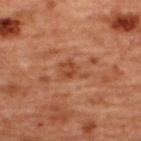Imaged during a routine full-body skin examination; the lesion was not biopsied and no histopathology is available. Measured at roughly 3.5 mm in maximum diameter. Cropped from a whole-body photographic skin survey; the tile spans about 15 mm. On the upper back. The lesion-visualizer software estimated a lesion area of about 5.5 mm² and an outline eccentricity of about 0.7 (0 = round, 1 = elongated). The analysis additionally found border irregularity of about 4.5 on a 0–10 scale, a color-variation rating of about 3/10, and radial color variation of about 1. The subject is a female aged 43 to 47. This is a cross-polarized tile.